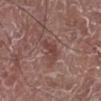The lesion-visualizer software estimated a border-irregularity index near 4/10.
A close-up tile cropped from a whole-body skin photograph, about 15 mm across.
From the right lower leg.
The lesion's longest dimension is about 3.5 mm.
This is a white-light tile.
A male patient, aged 73–77.